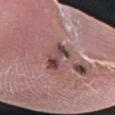acquisition = ~15 mm tile from a whole-body skin photo; lesion diameter = ≈3.5 mm; location = the left forearm; patient = female, aged approximately 70.